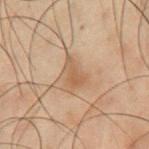follow-up = total-body-photography surveillance lesion; no biopsy
subject = male, roughly 50 years of age
body site = the chest
tile lighting = cross-polarized
imaging modality = total-body-photography crop, ~15 mm field of view
lesion size = ≈3.5 mm